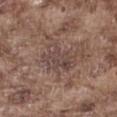biopsy status = imaged on a skin check; not biopsied | image = total-body-photography crop, ~15 mm field of view | patient = male, about 75 years old | lesion size = about 5 mm | site = the right thigh.